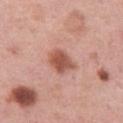Captured during whole-body skin photography for melanoma surveillance; the lesion was not biopsied. Cropped from a total-body skin-imaging series; the visible field is about 15 mm. This is a white-light tile. The subject is a female aged approximately 50. Automated image analysis of the tile measured a lesion area of about 7 mm², an eccentricity of roughly 0.7, and a symmetry-axis asymmetry near 0.25. And it measured a lesion color around L≈54 a*≈25 b*≈29 in CIELAB, a lesion–skin lightness drop of about 13, and a normalized lesion–skin contrast near 9. On the left thigh. Longest diameter approximately 3.5 mm.